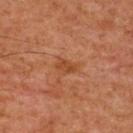Findings:
* biopsy status · catalogued during a skin exam; not biopsied
* subject · male, aged approximately 60
* image-analysis metrics · an area of roughly 4 mm², an eccentricity of roughly 0.85, and two-axis asymmetry of about 0.25; a mean CIELAB color near L≈41 a*≈24 b*≈34, roughly 7 lightness units darker than nearby skin, and a lesion-to-skin contrast of about 6 (normalized; higher = more distinct)
* body site · the front of the torso
* size · ~3 mm (longest diameter)
* acquisition · 15 mm crop, total-body photography
* illumination · cross-polarized illumination